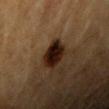Part of a total-body skin-imaging series; this lesion was reviewed on a skin check and was not flagged for biopsy.
The lesion-visualizer software estimated a lesion color around L≈16 a*≈15 b*≈19 in CIELAB, roughly 14 lightness units darker than nearby skin, and a normalized border contrast of about 17.5.
The tile uses cross-polarized illumination.
The subject is a male aged 83 or older.
The lesion is located on the right upper arm.
Cropped from a total-body skin-imaging series; the visible field is about 15 mm.